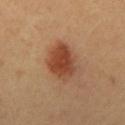Assessment:
Imaged during a routine full-body skin examination; the lesion was not biopsied and no histopathology is available.
Context:
Longest diameter approximately 5.5 mm. The subject is male. This is a cross-polarized tile. A roughly 15 mm field-of-view crop from a total-body skin photograph. The lesion is located on the mid back.